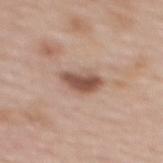Q: Is there a histopathology result?
A: total-body-photography surveillance lesion; no biopsy